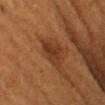<case>
<biopsy_status>not biopsied; imaged during a skin examination</biopsy_status>
<image>
  <source>total-body photography crop</source>
  <field_of_view_mm>15</field_of_view_mm>
</image>
<patient>
  <sex>female</sex>
  <age_approx>40</age_approx>
</patient>
<site>left thigh</site>
</case>